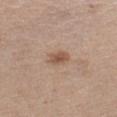<tbp_lesion>
<biopsy_status>not biopsied; imaged during a skin examination</biopsy_status>
<site>abdomen</site>
<lesion_size>
  <long_diameter_mm_approx>2.5</long_diameter_mm_approx>
</lesion_size>
<patient>
  <sex>female</sex>
  <age_approx>65</age_approx>
</patient>
<lighting>white-light</lighting>
<image>
  <source>total-body photography crop</source>
  <field_of_view_mm>15</field_of_view_mm>
</image>
</tbp_lesion>